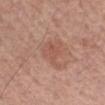workup: no biopsy performed (imaged during a skin exam)
anatomic site: the arm
image source: ~15 mm tile from a whole-body skin photo
automated lesion analysis: an eccentricity of roughly 0.65 and a shape-asymmetry score of about 0.55 (0 = symmetric); a border-irregularity index near 6/10 and peripheral color asymmetry of about 1; a detector confidence of about 100 out of 100 that the crop contains a lesion
subject: male, aged approximately 60
size: about 4 mm